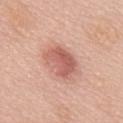This lesion was catalogued during total-body skin photography and was not selected for biopsy. Captured under white-light illumination. A female subject about 60 years old. Located on the back. A lesion tile, about 15 mm wide, cut from a 3D total-body photograph.